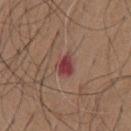Case summary:
* patient · male, about 65 years old
* anatomic site · the chest
* lesion diameter · ~3 mm (longest diameter)
* lighting · white-light illumination
* image source · ~15 mm tile from a whole-body skin photo
* image-analysis metrics · a normalized lesion–skin contrast near 9; a classifier nevus-likeness of about 0/100 and a lesion-detection confidence of about 100/100